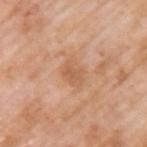<record>
<biopsy_status>not biopsied; imaged during a skin examination</biopsy_status>
<lesion_size>
  <long_diameter_mm_approx>2.5</long_diameter_mm_approx>
</lesion_size>
<patient>
  <sex>male</sex>
  <age_approx>60</age_approx>
</patient>
<site>left upper arm</site>
<automated_metrics>
  <area_mm2_approx>5.0</area_mm2_approx>
  <eccentricity>0.65</eccentricity>
  <shape_asymmetry>0.25</shape_asymmetry>
</automated_metrics>
<image>
  <source>total-body photography crop</source>
  <field_of_view_mm>15</field_of_view_mm>
</image>
<lighting>white-light</lighting>
</record>